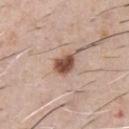No biopsy was performed on this lesion — it was imaged during a full skin examination and was not determined to be concerning. From the chest. A male patient aged 58 to 62. This is a white-light tile. Cropped from a total-body skin-imaging series; the visible field is about 15 mm. Approximately 3 mm at its widest. Automated image analysis of the tile measured an average lesion color of about L≈51 a*≈21 b*≈28 (CIELAB), a lesion–skin lightness drop of about 16, and a lesion-to-skin contrast of about 11 (normalized; higher = more distinct). It also reported internal color variation of about 4.5 on a 0–10 scale and peripheral color asymmetry of about 1.5.